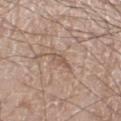Q: How large is the lesion?
A: ~2.5 mm (longest diameter)
Q: Illumination type?
A: white-light
Q: How was this image acquired?
A: 15 mm crop, total-body photography
Q: What is the anatomic site?
A: the left leg
Q: Patient demographics?
A: male, in their 80s